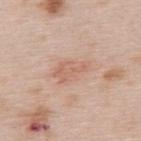Part of a total-body skin-imaging series; this lesion was reviewed on a skin check and was not flagged for biopsy. A 15 mm crop from a total-body photograph taken for skin-cancer surveillance. Automated image analysis of the tile measured an average lesion color of about L≈63 a*≈21 b*≈29 (CIELAB), a lesion–skin lightness drop of about 7, and a normalized lesion–skin contrast near 5. It also reported border irregularity of about 3.5 on a 0–10 scale and radial color variation of about 1. From the upper back. Longest diameter approximately 4.5 mm. This is a white-light tile. The subject is a female aged 63 to 67.